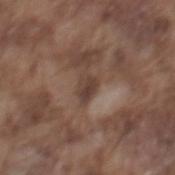| key | value |
|---|---|
| workup | imaged on a skin check; not biopsied |
| lighting | white-light illumination |
| patient | male, roughly 75 years of age |
| image source | total-body-photography crop, ~15 mm field of view |
| size | about 2.5 mm |
| location | the back |